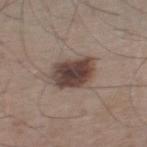Notes:
- biopsy status · catalogued during a skin exam; not biopsied
- illumination · white-light illumination
- acquisition · ~15 mm tile from a whole-body skin photo
- site · the left lower leg
- TBP lesion metrics · a lesion area of about 13 mm² and an outline eccentricity of about 0.7 (0 = round, 1 = elongated); an average lesion color of about L≈42 a*≈15 b*≈20 (CIELAB) and a normalized lesion–skin contrast near 11.5; a border-irregularity index near 2/10; a classifier nevus-likeness of about 90/100
- lesion size · ~4.5 mm (longest diameter)
- patient · male, approximately 60 years of age